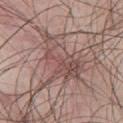This lesion was catalogued during total-body skin photography and was not selected for biopsy. A 15 mm crop from a total-body photograph taken for skin-cancer surveillance. On the upper back. The lesion-visualizer software estimated a lesion area of about 17 mm², an eccentricity of roughly 0.8, and two-axis asymmetry of about 0.45. It also reported a lesion color around L≈50 a*≈19 b*≈21 in CIELAB and a normalized border contrast of about 6. The software also gave a within-lesion color-variation index near 6/10 and a peripheral color-asymmetry measure near 2. The software also gave lesion-presence confidence of about 95/100. The tile uses white-light illumination. Longest diameter approximately 7 mm. A male patient, approximately 45 years of age.